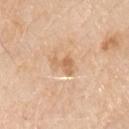lighting: white-light | subject: male, roughly 80 years of age | image-analysis metrics: a footprint of about 4.5 mm² and an outline eccentricity of about 0.75 (0 = round, 1 = elongated); an average lesion color of about L≈65 a*≈20 b*≈37 (CIELAB), about 9 CIELAB-L* units darker than the surrounding skin, and a lesion-to-skin contrast of about 6 (normalized; higher = more distinct); internal color variation of about 2.5 on a 0–10 scale and a peripheral color-asymmetry measure near 1 | diameter: ≈3 mm | site: the chest | image: ~15 mm crop, total-body skin-cancer survey.